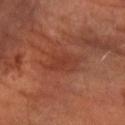{"biopsy_status": "not biopsied; imaged during a skin examination", "image": {"source": "total-body photography crop", "field_of_view_mm": 15}, "patient": {"sex": "male", "age_approx": 65}, "site": "left forearm"}